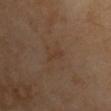Notes:
– workup — catalogued during a skin exam; not biopsied
– illumination — cross-polarized
– acquisition — ~15 mm crop, total-body skin-cancer survey
– anatomic site — the chest
– patient — male, aged approximately 55
– diameter — ≈2.5 mm
– automated metrics — an outline eccentricity of about 0.9 (0 = round, 1 = elongated) and two-axis asymmetry of about 0.4; border irregularity of about 4 on a 0–10 scale and peripheral color asymmetry of about 0